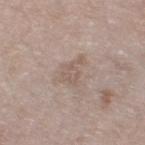* notes · total-body-photography surveillance lesion; no biopsy
* lighting · white-light illumination
* image-analysis metrics · a mean CIELAB color near L≈57 a*≈14 b*≈23, roughly 7 lightness units darker than nearby skin, and a lesion-to-skin contrast of about 5 (normalized; higher = more distinct); a classifier nevus-likeness of about 0/100 and a lesion-detection confidence of about 70/100
* size · ≈3.5 mm
* subject · female, aged approximately 75
* image source · ~15 mm crop, total-body skin-cancer survey
* location · the right thigh